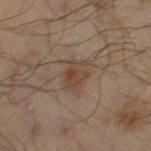Case summary:
- workup · total-body-photography surveillance lesion; no biopsy